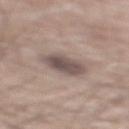<record>
  <biopsy_status>not biopsied; imaged during a skin examination</biopsy_status>
  <lesion_size>
    <long_diameter_mm_approx>5.0</long_diameter_mm_approx>
  </lesion_size>
  <lighting>white-light</lighting>
  <site>back</site>
  <image>
    <source>total-body photography crop</source>
    <field_of_view_mm>15</field_of_view_mm>
  </image>
  <patient>
    <sex>male</sex>
    <age_approx>60</age_approx>
  </patient>
</record>